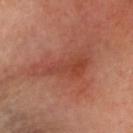<record>
<biopsy_status>not biopsied; imaged during a skin examination</biopsy_status>
<patient>
  <sex>female</sex>
  <age_approx>60</age_approx>
</patient>
<image>
  <source>total-body photography crop</source>
  <field_of_view_mm>15</field_of_view_mm>
</image>
<lesion_size>
  <long_diameter_mm_approx>7.0</long_diameter_mm_approx>
</lesion_size>
<lighting>cross-polarized</lighting>
<site>head or neck</site>
<automated_metrics>
  <area_mm2_approx>14.0</area_mm2_approx>
  <eccentricity>0.9</eccentricity>
  <shape_asymmetry>0.45</shape_asymmetry>
</automated_metrics>
</record>